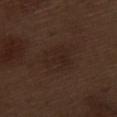Imaged during a routine full-body skin examination; the lesion was not biopsied and no histopathology is available.
Automated tile analysis of the lesion measured a within-lesion color-variation index near 2.5/10 and a peripheral color-asymmetry measure near 1. And it measured a classifier nevus-likeness of about 5/100 and a detector confidence of about 100 out of 100 that the crop contains a lesion.
Cropped from a whole-body photographic skin survey; the tile spans about 15 mm.
The subject is a male in their 70s.
Imaged with white-light lighting.
From the leg.
About 4.5 mm across.